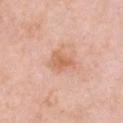<lesion>
  <biopsy_status>not biopsied; imaged during a skin examination</biopsy_status>
  <lighting>white-light</lighting>
  <patient>
    <sex>female</sex>
    <age_approx>45</age_approx>
  </patient>
  <site>front of the torso</site>
  <automated_metrics>
    <cielab_L>64</cielab_L>
    <cielab_a>24</cielab_a>
    <cielab_b>35</cielab_b>
    <vs_skin_darker_L>9.0</vs_skin_darker_L>
    <vs_skin_contrast_norm>7.0</vs_skin_contrast_norm>
    <border_irregularity_0_10>3.0</border_irregularity_0_10>
    <peripheral_color_asymmetry>0.5</peripheral_color_asymmetry>
  </automated_metrics>
  <image>
    <source>total-body photography crop</source>
    <field_of_view_mm>15</field_of_view_mm>
  </image>
  <lesion_size>
    <long_diameter_mm_approx>3.0</long_diameter_mm_approx>
  </lesion_size>
</lesion>